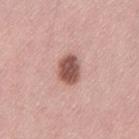Clinical impression: Captured during whole-body skin photography for melanoma surveillance; the lesion was not biopsied. Acquisition and patient details: Automated image analysis of the tile measured an automated nevus-likeness rating near 95 out of 100 and lesion-presence confidence of about 100/100. The lesion's longest dimension is about 3.5 mm. The patient is a female aged 48 to 52. The lesion is on the back. A 15 mm close-up extracted from a 3D total-body photography capture.